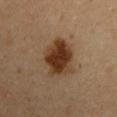notes: total-body-photography surveillance lesion; no biopsy | image: total-body-photography crop, ~15 mm field of view | diameter: ≈5 mm | location: the left upper arm | patient: female, aged approximately 45 | lighting: cross-polarized illumination.